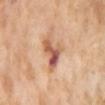Automated image analysis of the tile measured a lesion area of about 9 mm² and a shape eccentricity near 0.7. The analysis additionally found a border-irregularity index near 5.5/10, a within-lesion color-variation index near 10/10, and a peripheral color-asymmetry measure near 4.
Cropped from a total-body skin-imaging series; the visible field is about 15 mm.
The lesion is on the abdomen.
A female subject approximately 55 years of age.